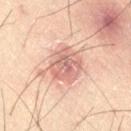Assessment: Captured during whole-body skin photography for melanoma surveillance; the lesion was not biopsied. Background: A male subject, roughly 50 years of age. Measured at roughly 4.5 mm in maximum diameter. Located on the right thigh. A region of skin cropped from a whole-body photographic capture, roughly 15 mm wide. This is a cross-polarized tile.